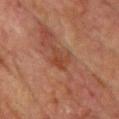{"biopsy_status": "not biopsied; imaged during a skin examination", "image": {"source": "total-body photography crop", "field_of_view_mm": 15}, "automated_metrics": {"eccentricity": 0.7, "shape_asymmetry": 0.4, "cielab_L": 36, "cielab_a": 22, "cielab_b": 28, "vs_skin_darker_L": 6.0, "vs_skin_contrast_norm": 6.0}, "patient": {"sex": "male", "age_approx": 75}, "site": "front of the torso", "lighting": "cross-polarized"}